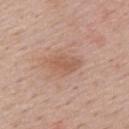Imaged during a routine full-body skin examination; the lesion was not biopsied and no histopathology is available.
A male subject, roughly 55 years of age.
On the upper back.
This image is a 15 mm lesion crop taken from a total-body photograph.